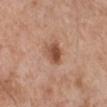Automated tile analysis of the lesion measured a shape eccentricity near 0.45 and a symmetry-axis asymmetry near 0.25. And it measured a mean CIELAB color near L≈51 a*≈23 b*≈31 and about 12 CIELAB-L* units darker than the surrounding skin. Located on the leg. The subject is a male aged approximately 80. A 15 mm close-up extracted from a 3D total-body photography capture.